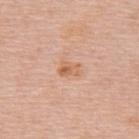This lesion was catalogued during total-body skin photography and was not selected for biopsy. A female patient, approximately 50 years of age. This image is a 15 mm lesion crop taken from a total-body photograph. Located on the upper back.